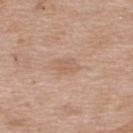biopsy status: total-body-photography surveillance lesion; no biopsy | tile lighting: white-light illumination | acquisition: total-body-photography crop, ~15 mm field of view | body site: the upper back | subject: female, aged 38 to 42 | lesion diameter: ≈3 mm.